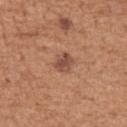Captured during whole-body skin photography for melanoma surveillance; the lesion was not biopsied. A female patient, approximately 55 years of age. On the arm. The recorded lesion diameter is about 2.5 mm. A 15 mm close-up extracted from a 3D total-body photography capture.